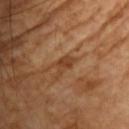notes: imaged on a skin check; not biopsied
site: the chest
lighting: cross-polarized
diameter: ~3 mm (longest diameter)
image source: 15 mm crop, total-body photography
automated lesion analysis: a lesion area of about 3.5 mm², a shape eccentricity near 0.8, and a symmetry-axis asymmetry near 0.35; a lesion color around L≈40 a*≈21 b*≈34 in CIELAB, about 8 CIELAB-L* units darker than the surrounding skin, and a normalized lesion–skin contrast near 7
patient: male, roughly 60 years of age